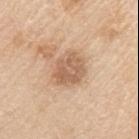notes: catalogued during a skin exam; not biopsied
lesion size: ≈4 mm
acquisition: total-body-photography crop, ~15 mm field of view
lighting: white-light illumination
patient: male, about 60 years old
anatomic site: the right upper arm